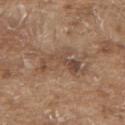Clinical impression:
No biopsy was performed on this lesion — it was imaged during a full skin examination and was not determined to be concerning.
Image and clinical context:
An algorithmic analysis of the crop reported an area of roughly 12 mm², an outline eccentricity of about 0.9 (0 = round, 1 = elongated), and a shape-asymmetry score of about 0.6 (0 = symmetric). The software also gave border irregularity of about 9.5 on a 0–10 scale and peripheral color asymmetry of about 2.5. The software also gave a classifier nevus-likeness of about 0/100 and a detector confidence of about 85 out of 100 that the crop contains a lesion. Captured under white-light illumination. The lesion's longest dimension is about 7 mm. A roughly 15 mm field-of-view crop from a total-body skin photograph. On the upper back. A male patient approximately 80 years of age.